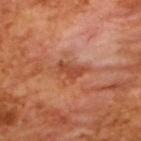<record>
  <biopsy_status>not biopsied; imaged during a skin examination</biopsy_status>
  <automated_metrics>
    <cielab_L>46</cielab_L>
    <cielab_a>29</cielab_a>
    <cielab_b>35</cielab_b>
    <vs_skin_darker_L>10.0</vs_skin_darker_L>
    <peripheral_color_asymmetry>1.0</peripheral_color_asymmetry>
    <lesion_detection_confidence_0_100>100</lesion_detection_confidence_0_100>
  </automated_metrics>
  <lighting>cross-polarized</lighting>
  <image>
    <source>total-body photography crop</source>
    <field_of_view_mm>15</field_of_view_mm>
  </image>
  <patient>
    <sex>male</sex>
    <age_approx>65</age_approx>
  </patient>
  <lesion_size>
    <long_diameter_mm_approx>3.5</long_diameter_mm_approx>
  </lesion_size>
</record>